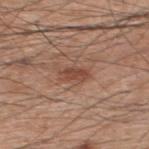location — the upper back
tile lighting — white-light illumination
patient — male, about 60 years old
TBP lesion metrics — a lesion color around L≈47 a*≈22 b*≈28 in CIELAB and about 9 CIELAB-L* units darker than the surrounding skin; a color-variation rating of about 2/10
lesion diameter — ≈3.5 mm
image source — 15 mm crop, total-body photography